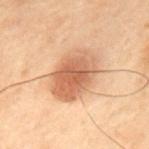| feature | finding |
|---|---|
| biopsy status | no biopsy performed (imaged during a skin exam) |
| body site | the upper back |
| lesion diameter | about 6 mm |
| image source | 15 mm crop, total-body photography |
| patient | male, aged approximately 70 |